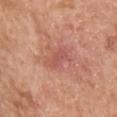Assessment: The lesion was photographed on a routine skin check and not biopsied; there is no pathology result. Clinical summary: Automated tile analysis of the lesion measured an area of roughly 4.5 mm², an eccentricity of roughly 0.95, and a shape-asymmetry score of about 0.25 (0 = symmetric). It also reported an automated nevus-likeness rating near 5 out of 100 and a detector confidence of about 100 out of 100 that the crop contains a lesion. From the chest. The patient is a male aged approximately 60. This is a white-light tile. A close-up tile cropped from a whole-body skin photograph, about 15 mm across. Approximately 3.5 mm at its widest.